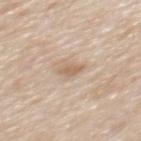Impression:
Part of a total-body skin-imaging series; this lesion was reviewed on a skin check and was not flagged for biopsy.